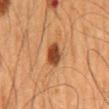<tbp_lesion>
<biopsy_status>not biopsied; imaged during a skin examination</biopsy_status>
<patient>
  <sex>male</sex>
  <age_approx>65</age_approx>
</patient>
<image>
  <source>total-body photography crop</source>
  <field_of_view_mm>15</field_of_view_mm>
</image>
<site>mid back</site>
</tbp_lesion>